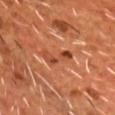Part of a total-body skin-imaging series; this lesion was reviewed on a skin check and was not flagged for biopsy. The lesion is on the chest. A male patient, aged around 55. A 15 mm close-up extracted from a 3D total-body photography capture. Captured under cross-polarized illumination.